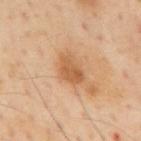• biopsy status · catalogued during a skin exam; not biopsied
• size · ≈3.5 mm
• location · the mid back
• image · ~15 mm crop, total-body skin-cancer survey
• subject · male, aged approximately 55